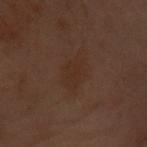  biopsy_status: not biopsied; imaged during a skin examination
  lighting: cross-polarized
  site: front of the torso
  patient:
    sex: female
    age_approx: 60
  image:
    source: total-body photography crop
    field_of_view_mm: 15
  lesion_size:
    long_diameter_mm_approx: 3.0
  automated_metrics:
    cielab_L: 24
    cielab_a: 15
    cielab_b: 21
    vs_skin_darker_L: 3.0
    vs_skin_contrast_norm: 4.5
    nevus_likeness_0_100: 0
    lesion_detection_confidence_0_100: 100